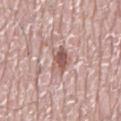Q: Is there a histopathology result?
A: total-body-photography surveillance lesion; no biopsy
Q: What are the patient's age and sex?
A: male, about 75 years old
Q: What did automated image analysis measure?
A: a lesion color around L≈57 a*≈20 b*≈23 in CIELAB and about 12 CIELAB-L* units darker than the surrounding skin
Q: Lesion location?
A: the mid back
Q: What kind of image is this?
A: 15 mm crop, total-body photography
Q: What is the lesion's diameter?
A: ~4 mm (longest diameter)